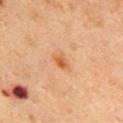This lesion was catalogued during total-body skin photography and was not selected for biopsy.
The lesion is located on the front of the torso.
The patient is a male about 75 years old.
The tile uses cross-polarized illumination.
A 15 mm close-up tile from a total-body photography series done for melanoma screening.
The recorded lesion diameter is about 2.5 mm.
An algorithmic analysis of the crop reported a footprint of about 3.5 mm², a shape eccentricity near 0.75, and a shape-asymmetry score of about 0.35 (0 = symmetric). It also reported about 7 CIELAB-L* units darker than the surrounding skin and a normalized lesion–skin contrast near 6.5. The analysis additionally found an automated nevus-likeness rating near 65 out of 100 and a detector confidence of about 100 out of 100 that the crop contains a lesion.